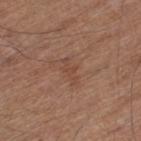Recorded during total-body skin imaging; not selected for excision or biopsy. A lesion tile, about 15 mm wide, cut from a 3D total-body photograph. The recorded lesion diameter is about 3.5 mm. On the left thigh. A male subject roughly 65 years of age.